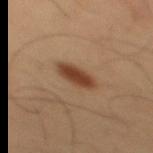Recorded during total-body skin imaging; not selected for excision or biopsy.
A male subject approximately 55 years of age.
A 15 mm crop from a total-body photograph taken for skin-cancer surveillance.
Imaged with cross-polarized lighting.
The lesion-visualizer software estimated a symmetry-axis asymmetry near 0.2. The software also gave a border-irregularity rating of about 2.5/10, internal color variation of about 2 on a 0–10 scale, and peripheral color asymmetry of about 0.5. The analysis additionally found a classifier nevus-likeness of about 100/100 and a lesion-detection confidence of about 100/100.
On the mid back.